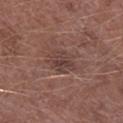subject: male, roughly 75 years of age | image source: 15 mm crop, total-body photography | site: the right lower leg.